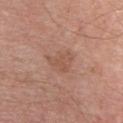Notes:
- notes · total-body-photography surveillance lesion; no biopsy
- patient · male, aged 53 to 57
- anatomic site · the chest
- illumination · white-light illumination
- diameter · ~3.5 mm (longest diameter)
- acquisition · total-body-photography crop, ~15 mm field of view
- automated metrics · border irregularity of about 5 on a 0–10 scale and a within-lesion color-variation index near 1.5/10